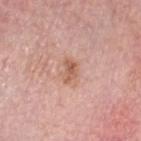Q: Lesion location?
A: the head or neck
Q: What did automated image analysis measure?
A: an area of roughly 4 mm², an eccentricity of roughly 0.8, and a symmetry-axis asymmetry near 0.35
Q: Illumination type?
A: white-light illumination
Q: What are the patient's age and sex?
A: male, aged around 60
Q: What kind of image is this?
A: ~15 mm crop, total-body skin-cancer survey
Q: Lesion size?
A: ≈3 mm